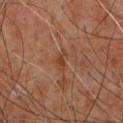From the upper back.
The recorded lesion diameter is about 2.5 mm.
Automated tile analysis of the lesion measured a shape eccentricity near 0.85 and a symmetry-axis asymmetry near 0.5. The software also gave an average lesion color of about L≈37 a*≈21 b*≈30 (CIELAB), a lesion–skin lightness drop of about 6, and a lesion-to-skin contrast of about 6.5 (normalized; higher = more distinct). It also reported a lesion-detection confidence of about 95/100.
A male subject aged around 60.
A close-up tile cropped from a whole-body skin photograph, about 15 mm across.